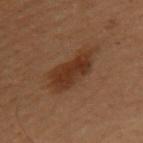Q: Was this lesion biopsied?
A: no biopsy performed (imaged during a skin exam)
Q: Patient demographics?
A: female, about 50 years old
Q: Lesion location?
A: the left upper arm
Q: Illumination type?
A: cross-polarized illumination
Q: What kind of image is this?
A: ~15 mm crop, total-body skin-cancer survey
Q: How large is the lesion?
A: ≈5.5 mm